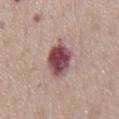No biopsy was performed on this lesion — it was imaged during a full skin examination and was not determined to be concerning. A male patient, approximately 75 years of age. The lesion is on the chest. A lesion tile, about 15 mm wide, cut from a 3D total-body photograph. The lesion's longest dimension is about 5.5 mm.